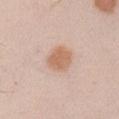Clinical impression:
Captured during whole-body skin photography for melanoma surveillance; the lesion was not biopsied.
Acquisition and patient details:
Cropped from a total-body skin-imaging series; the visible field is about 15 mm. The subject is a male aged 28–32. Approximately 3.5 mm at its widest. Captured under white-light illumination. From the arm.